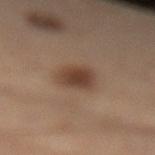Automated image analysis of the tile measured an area of roughly 7.5 mm² and two-axis asymmetry of about 0.15. It also reported a border-irregularity rating of about 2/10, a within-lesion color-variation index near 3.5/10, and peripheral color asymmetry of about 1.
A lesion tile, about 15 mm wide, cut from a 3D total-body photograph.
Approximately 3 mm at its widest.
From the leg.
Captured under cross-polarized illumination.
The patient is a male in their 50s.